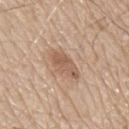location = the mid back
illumination = white-light illumination
subject = male, aged 78 to 82
image source = ~15 mm crop, total-body skin-cancer survey